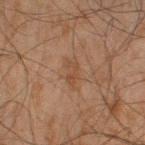<tbp_lesion>
  <biopsy_status>not biopsied; imaged during a skin examination</biopsy_status>
  <automated_metrics>
    <nevus_likeness_0_100>0</nevus_likeness_0_100>
    <lesion_detection_confidence_0_100>100</lesion_detection_confidence_0_100>
  </automated_metrics>
  <image>
    <source>total-body photography crop</source>
    <field_of_view_mm>15</field_of_view_mm>
  </image>
  <site>right lower leg</site>
  <patient>
    <sex>male</sex>
    <age_approx>45</age_approx>
  </patient>
  <lesion_size>
    <long_diameter_mm_approx>3.5</long_diameter_mm_approx>
  </lesion_size>
  <lighting>cross-polarized</lighting>
</tbp_lesion>